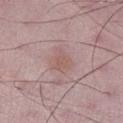No biopsy was performed on this lesion — it was imaged during a full skin examination and was not determined to be concerning.
Captured under white-light illumination.
From the right lower leg.
Automated image analysis of the tile measured an automated nevus-likeness rating near 15 out of 100 and a detector confidence of about 100 out of 100 that the crop contains a lesion.
A male patient, aged 48 to 52.
The lesion's longest dimension is about 3.5 mm.
This image is a 15 mm lesion crop taken from a total-body photograph.